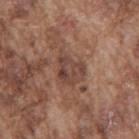<record>
<biopsy_status>not biopsied; imaged during a skin examination</biopsy_status>
<patient>
  <sex>male</sex>
  <age_approx>75</age_approx>
</patient>
<lesion_size>
  <long_diameter_mm_approx>4.0</long_diameter_mm_approx>
</lesion_size>
<automated_metrics>
  <area_mm2_approx>9.0</area_mm2_approx>
  <eccentricity>0.65</eccentricity>
  <shape_asymmetry>0.4</shape_asymmetry>
  <cielab_L>43</cielab_L>
  <cielab_a>19</cielab_a>
  <cielab_b>25</cielab_b>
  <vs_skin_contrast_norm>7.5</vs_skin_contrast_norm>
  <border_irregularity_0_10>4.0</border_irregularity_0_10>
  <color_variation_0_10>5.0</color_variation_0_10>
  <peripheral_color_asymmetry>1.5</peripheral_color_asymmetry>
  <nevus_likeness_0_100>0</nevus_likeness_0_100>
</automated_metrics>
<site>back</site>
<lighting>white-light</lighting>
<image>
  <source>total-body photography crop</source>
  <field_of_view_mm>15</field_of_view_mm>
</image>
</record>